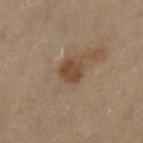Findings:
• notes · total-body-photography surveillance lesion; no biopsy
• tile lighting · cross-polarized
• patient · female, in their 50s
• anatomic site · the leg
• automated metrics · a lesion color around L≈37 a*≈15 b*≈25 in CIELAB, a lesion–skin lightness drop of about 8, and a normalized border contrast of about 8; a border-irregularity rating of about 1.5/10 and a color-variation rating of about 2/10; a nevus-likeness score of about 80/100 and a detector confidence of about 100 out of 100 that the crop contains a lesion
• image · 15 mm crop, total-body photography